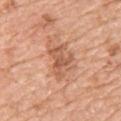Q: Is there a histopathology result?
A: total-body-photography surveillance lesion; no biopsy
Q: Where on the body is the lesion?
A: the chest
Q: How was this image acquired?
A: 15 mm crop, total-body photography
Q: Illumination type?
A: white-light illumination
Q: Who is the patient?
A: female, in their mid- to late 40s
Q: Lesion size?
A: ≈4.5 mm